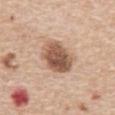Imaged during a routine full-body skin examination; the lesion was not biopsied and no histopathology is available.
A male patient, aged around 70.
This image is a 15 mm lesion crop taken from a total-body photograph.
The lesion's longest dimension is about 4.5 mm.
On the abdomen.
Automated image analysis of the tile measured a lesion area of about 13 mm², an eccentricity of roughly 0.55, and two-axis asymmetry of about 0.15. And it measured a within-lesion color-variation index near 6/10 and peripheral color asymmetry of about 2.
The tile uses white-light illumination.